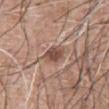A close-up tile cropped from a whole-body skin photograph, about 15 mm across. A male patient, aged around 60. From the front of the torso.